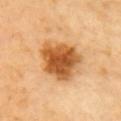Context:
From the upper back. The subject is a female approximately 60 years of age. Automated image analysis of the tile measured a border-irregularity index near 3/10, a color-variation rating of about 6/10, and radial color variation of about 1.5. And it measured an automated nevus-likeness rating near 95 out of 100 and a lesion-detection confidence of about 100/100. About 5.5 mm across. A 15 mm close-up tile from a total-body photography series done for melanoma screening. Imaged with cross-polarized lighting.